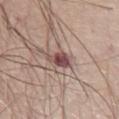– follow-up — imaged on a skin check; not biopsied
– tile lighting — white-light
– acquisition — ~15 mm tile from a whole-body skin photo
– patient — male, approximately 70 years of age
– location — the chest